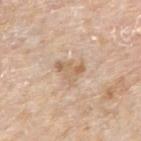workup = total-body-photography surveillance lesion; no biopsy
acquisition = ~15 mm tile from a whole-body skin photo
patient = male, aged 73–77
site = the right upper arm
size = about 3.5 mm
TBP lesion metrics = a border-irregularity index near 5/10, a color-variation rating of about 4/10, and a peripheral color-asymmetry measure near 1.5; a nevus-likeness score of about 0/100 and a lesion-detection confidence of about 100/100